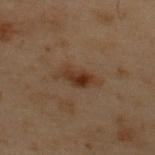No biopsy was performed on this lesion — it was imaged during a full skin examination and was not determined to be concerning. A roughly 15 mm field-of-view crop from a total-body skin photograph. This is a cross-polarized tile. About 4.5 mm across. Automated image analysis of the tile measured an eccentricity of roughly 0.85 and a symmetry-axis asymmetry near 0.25. And it measured an average lesion color of about L≈28 a*≈14 b*≈24 (CIELAB) and a normalized border contrast of about 7.5. The analysis additionally found internal color variation of about 5 on a 0–10 scale and peripheral color asymmetry of about 1.5. The analysis additionally found a classifier nevus-likeness of about 80/100 and lesion-presence confidence of about 100/100. A male subject, aged approximately 55. The lesion is on the upper back.